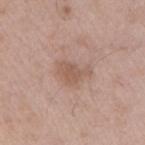  biopsy_status: not biopsied; imaged during a skin examination
  lesion_size:
    long_diameter_mm_approx: 3.5
  site: right upper arm
  patient:
    sex: male
    age_approx: 40
  automated_metrics:
    cielab_L: 56
    cielab_a: 19
    cielab_b: 27
    vs_skin_darker_L: 8.0
    vs_skin_contrast_norm: 6.0
    border_irregularity_0_10: 4.5
    color_variation_0_10: 1.5
    nevus_likeness_0_100: 10
    lesion_detection_confidence_0_100: 100
  lighting: white-light
  image:
    source: total-body photography crop
    field_of_view_mm: 15